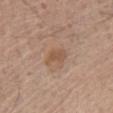biopsy status: imaged on a skin check; not biopsied
lighting: white-light
lesion diameter: ≈2.5 mm
subject: male, about 80 years old
image: total-body-photography crop, ~15 mm field of view
anatomic site: the chest